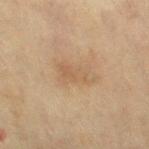{
  "biopsy_status": "not biopsied; imaged during a skin examination",
  "image": {
    "source": "total-body photography crop",
    "field_of_view_mm": 15
  },
  "lighting": "cross-polarized",
  "site": "right thigh",
  "automated_metrics": {
    "area_mm2_approx": 8.5,
    "eccentricity": 0.85,
    "shape_asymmetry": 0.3,
    "cielab_L": 51,
    "cielab_a": 14,
    "cielab_b": 30,
    "vs_skin_darker_L": 6.0,
    "vs_skin_contrast_norm": 5.0,
    "border_irregularity_0_10": 3.5,
    "color_variation_0_10": 2.5,
    "peripheral_color_asymmetry": 1.0,
    "nevus_likeness_0_100": 0,
    "lesion_detection_confidence_0_100": 100
  },
  "lesion_size": {
    "long_diameter_mm_approx": 4.5
  },
  "patient": {
    "sex": "female",
    "age_approx": 55
  }
}